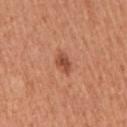  patient:
    sex: female
    age_approx: 50
  lesion_size:
    long_diameter_mm_approx: 2.5
  image:
    source: total-body photography crop
    field_of_view_mm: 15
  site: right upper arm
  lighting: white-light